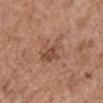Approximately 5 mm at its widest. A female patient roughly 65 years of age. This image is a 15 mm lesion crop taken from a total-body photograph. The lesion is on the right upper arm. Captured under white-light illumination. Automated tile analysis of the lesion measured an average lesion color of about L≈52 a*≈21 b*≈29 (CIELAB), a lesion–skin lightness drop of about 7, and a normalized border contrast of about 5. The software also gave a border-irregularity index near 4/10, a color-variation rating of about 6/10, and a peripheral color-asymmetry measure near 2.